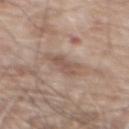| feature | finding |
|---|---|
| notes | catalogued during a skin exam; not biopsied |
| subject | male, aged approximately 80 |
| body site | the mid back |
| acquisition | total-body-photography crop, ~15 mm field of view |